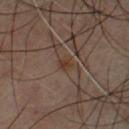{"biopsy_status": "not biopsied; imaged during a skin examination", "image": {"source": "total-body photography crop", "field_of_view_mm": 15}, "lesion_size": {"long_diameter_mm_approx": 1.5}, "patient": {"sex": "male", "age_approx": 45}, "site": "chest"}